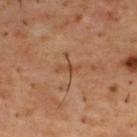Case summary:
– biopsy status: no biopsy performed (imaged during a skin exam)
– image-analysis metrics: a lesion color around L≈46 a*≈22 b*≈33 in CIELAB and a normalized lesion–skin contrast near 5.5; a border-irregularity rating of about 5.5/10 and a peripheral color-asymmetry measure near 0; an automated nevus-likeness rating near 0 out of 100
– patient: male, aged around 55
– lesion size: ≈2.5 mm
– site: the upper back
– acquisition: ~15 mm tile from a whole-body skin photo